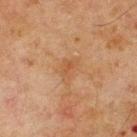The lesion was photographed on a routine skin check and not biopsied; there is no pathology result.
The lesion-visualizer software estimated a shape eccentricity near 0.75 and two-axis asymmetry of about 0.4. The software also gave about 5 CIELAB-L* units darker than the surrounding skin and a lesion-to-skin contrast of about 4.5 (normalized; higher = more distinct). It also reported a border-irregularity index near 4/10. The software also gave a classifier nevus-likeness of about 0/100.
Longest diameter approximately 3 mm.
Imaged with cross-polarized lighting.
Located on the upper back.
A 15 mm crop from a total-body photograph taken for skin-cancer surveillance.
A male patient, aged approximately 70.